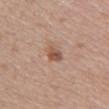This lesion was catalogued during total-body skin photography and was not selected for biopsy. Automated tile analysis of the lesion measured a mean CIELAB color near L≈52 a*≈21 b*≈29 and roughly 11 lightness units darker than nearby skin. The software also gave a nevus-likeness score of about 60/100 and a detector confidence of about 100 out of 100 that the crop contains a lesion. A female patient, about 50 years old. About 2.5 mm across. Cropped from a whole-body photographic skin survey; the tile spans about 15 mm. The tile uses white-light illumination. From the chest.